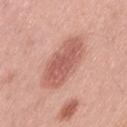<case>
  <biopsy_status>not biopsied; imaged during a skin examination</biopsy_status>
  <lesion_size>
    <long_diameter_mm_approx>7.0</long_diameter_mm_approx>
  </lesion_size>
  <patient>
    <sex>female</sex>
    <age_approx>40</age_approx>
  </patient>
  <lighting>white-light</lighting>
  <automated_metrics>
    <nevus_likeness_0_100>50</nevus_likeness_0_100>
  </automated_metrics>
  <site>lower back</site>
  <image>
    <source>total-body photography crop</source>
    <field_of_view_mm>15</field_of_view_mm>
  </image>
</case>